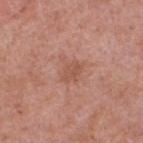{"biopsy_status": "not biopsied; imaged during a skin examination", "patient": {"sex": "female", "age_approx": 40}, "image": {"source": "total-body photography crop", "field_of_view_mm": 15}, "site": "left lower leg", "lesion_size": {"long_diameter_mm_approx": 2.5}, "lighting": "white-light"}